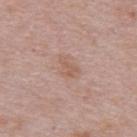<record>
  <biopsy_status>not biopsied; imaged during a skin examination</biopsy_status>
  <site>upper back</site>
  <lighting>white-light</lighting>
  <lesion_size>
    <long_diameter_mm_approx>3.0</long_diameter_mm_approx>
  </lesion_size>
  <patient>
    <sex>female</sex>
    <age_approx>65</age_approx>
  </patient>
  <image>
    <source>total-body photography crop</source>
    <field_of_view_mm>15</field_of_view_mm>
  </image>
</record>